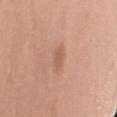  biopsy_status: not biopsied; imaged during a skin examination
  image:
    source: total-body photography crop
    field_of_view_mm: 15
  lesion_size:
    long_diameter_mm_approx: 2.5
  patient:
    sex: female
    age_approx: 65
  lighting: white-light
  automated_metrics:
    eccentricity: 0.8
    shape_asymmetry: 0.35
    cielab_L: 58
    cielab_a: 23
    cielab_b: 32
    vs_skin_darker_L: 8.0
    border_irregularity_0_10: 3.5
    color_variation_0_10: 2.0
  site: right upper arm